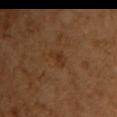  biopsy_status: not biopsied; imaged during a skin examination
  site: front of the torso
  patient:
    sex: female
    age_approx: 55
  image:
    source: total-body photography crop
    field_of_view_mm: 15
  automated_metrics:
    cielab_L: 32
    cielab_a: 20
    cielab_b: 31
    vs_skin_darker_L: 6.0
    vs_skin_contrast_norm: 5.5
    border_irregularity_0_10: 5.0
    color_variation_0_10: 0.0
    peripheral_color_asymmetry: 0.0
    lesion_detection_confidence_0_100: 100
  lesion_size:
    long_diameter_mm_approx: 2.5
  lighting: cross-polarized